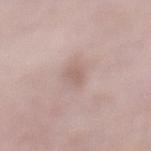The lesion was tiled from a total-body skin photograph and was not biopsied. The lesion is on the leg. Measured at roughly 2.5 mm in maximum diameter. Automated tile analysis of the lesion measured a footprint of about 2.5 mm², an outline eccentricity of about 0.85 (0 = round, 1 = elongated), and a shape-asymmetry score of about 0.3 (0 = symmetric). And it measured a border-irregularity index near 3/10, a color-variation rating of about 0.5/10, and peripheral color asymmetry of about 0. It also reported an automated nevus-likeness rating near 0 out of 100 and lesion-presence confidence of about 95/100. This image is a 15 mm lesion crop taken from a total-body photograph. Captured under white-light illumination. A female patient, roughly 70 years of age.